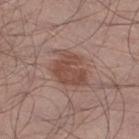Recorded during total-body skin imaging; not selected for excision or biopsy. A roughly 15 mm field-of-view crop from a total-body skin photograph. The patient is a male in their mid- to late 50s. The lesion is located on the right lower leg.